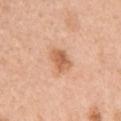notes: catalogued during a skin exam; not biopsied | body site: the left upper arm | tile lighting: white-light | image source: ~15 mm tile from a whole-body skin photo | subject: female, roughly 60 years of age.